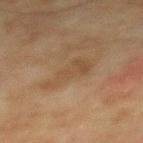Q: Is there a histopathology result?
A: catalogued during a skin exam; not biopsied
Q: What is the anatomic site?
A: the mid back
Q: What did automated image analysis measure?
A: an average lesion color of about L≈40 a*≈14 b*≈28 (CIELAB); a border-irregularity index near 5.5/10, a within-lesion color-variation index near 2/10, and a peripheral color-asymmetry measure near 0.5
Q: Illumination type?
A: cross-polarized illumination
Q: Patient demographics?
A: male, aged approximately 75
Q: What is the imaging modality?
A: ~15 mm crop, total-body skin-cancer survey